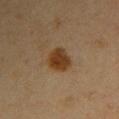This lesion was catalogued during total-body skin photography and was not selected for biopsy.
A lesion tile, about 15 mm wide, cut from a 3D total-body photograph.
This is a cross-polarized tile.
Automated tile analysis of the lesion measured a lesion color around L≈32 a*≈16 b*≈30 in CIELAB and a normalized border contrast of about 11. The analysis additionally found a lesion-detection confidence of about 100/100.
The subject is a female aged approximately 45.
Located on the right upper arm.